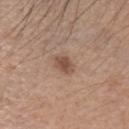biopsy_status: not biopsied; imaged during a skin examination
automated_metrics:
  eccentricity: 0.6
  shape_asymmetry: 0.2
patient:
  sex: female
  age_approx: 65
site: head or neck
lesion_size:
  long_diameter_mm_approx: 2.5
image:
  source: total-body photography crop
  field_of_view_mm: 15